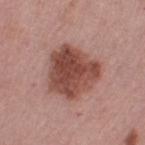Clinical impression:
Part of a total-body skin-imaging series; this lesion was reviewed on a skin check and was not flagged for biopsy.
Acquisition and patient details:
A female subject roughly 65 years of age. On the right thigh. A lesion tile, about 15 mm wide, cut from a 3D total-body photograph.